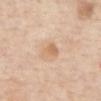<record>
<biopsy_status>not biopsied; imaged during a skin examination</biopsy_status>
<site>front of the torso</site>
<patient>
  <sex>female</sex>
  <age_approx>65</age_approx>
</patient>
<lighting>white-light</lighting>
<automated_metrics>
  <area_mm2_approx>5.0</area_mm2_approx>
  <eccentricity>0.65</eccentricity>
  <shape_asymmetry>0.3</shape_asymmetry>
  <nevus_likeness_0_100>25</nevus_likeness_0_100>
  <lesion_detection_confidence_0_100>100</lesion_detection_confidence_0_100>
</automated_metrics>
<lesion_size>
  <long_diameter_mm_approx>3.0</long_diameter_mm_approx>
</lesion_size>
<image>
  <source>total-body photography crop</source>
  <field_of_view_mm>15</field_of_view_mm>
</image>
</record>